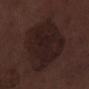Assessment:
The lesion was photographed on a routine skin check and not biopsied; there is no pathology result.
Clinical summary:
A male subject about 70 years old. The lesion-visualizer software estimated a lesion area of about 42 mm², an outline eccentricity of about 0.7 (0 = round, 1 = elongated), and a shape-asymmetry score of about 0.2 (0 = symmetric). The analysis additionally found a border-irregularity rating of about 2/10, a within-lesion color-variation index near 3/10, and radial color variation of about 1. Captured under white-light illumination. The lesion is located on the left lower leg. A region of skin cropped from a whole-body photographic capture, roughly 15 mm wide.